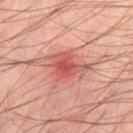Impression:
The lesion was tiled from a total-body skin photograph and was not biopsied.
Clinical summary:
A 15 mm crop from a total-body photograph taken for skin-cancer surveillance. The lesion is on the leg. A male patient, aged 43 to 47. Captured under cross-polarized illumination. Measured at roughly 5.5 mm in maximum diameter.